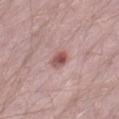patient:
  sex: male
  age_approx: 50
site: right thigh
image:
  source: total-body photography crop
  field_of_view_mm: 15
lesion_size:
  long_diameter_mm_approx: 2.5